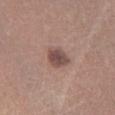Q: Was a biopsy performed?
A: no biopsy performed (imaged during a skin exam)
Q: How was the tile lit?
A: white-light illumination
Q: Automated lesion metrics?
A: an area of roughly 6 mm², an outline eccentricity of about 0.5 (0 = round, 1 = elongated), and a symmetry-axis asymmetry near 0.2; about 12 CIELAB-L* units darker than the surrounding skin and a normalized border contrast of about 9; a border-irregularity index near 2/10, a within-lesion color-variation index near 2.5/10, and a peripheral color-asymmetry measure near 0.5
Q: Patient demographics?
A: female, about 55 years old
Q: Lesion size?
A: ≈3 mm
Q: How was this image acquired?
A: 15 mm crop, total-body photography
Q: Where on the body is the lesion?
A: the right lower leg